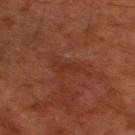Notes:
• workup — total-body-photography surveillance lesion; no biopsy
• image source — 15 mm crop, total-body photography
• tile lighting — cross-polarized
• site — the right thigh
• diameter — about 4.5 mm
• subject — male, aged 78 to 82